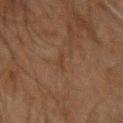<record>
  <biopsy_status>not biopsied; imaged during a skin examination</biopsy_status>
  <lesion_size>
    <long_diameter_mm_approx>2.5</long_diameter_mm_approx>
  </lesion_size>
  <image>
    <source>total-body photography crop</source>
    <field_of_view_mm>15</field_of_view_mm>
  </image>
  <patient>
    <sex>male</sex>
    <age_approx>60</age_approx>
  </patient>
  <site>left arm</site>
  <lighting>cross-polarized</lighting>
</record>